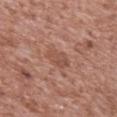Q: Was this lesion biopsied?
A: no biopsy performed (imaged during a skin exam)
Q: What did automated image analysis measure?
A: a mean CIELAB color near L≈51 a*≈23 b*≈28 and a lesion-to-skin contrast of about 5.5 (normalized; higher = more distinct); internal color variation of about 2.5 on a 0–10 scale and peripheral color asymmetry of about 1; a detector confidence of about 100 out of 100 that the crop contains a lesion
Q: What is the imaging modality?
A: total-body-photography crop, ~15 mm field of view
Q: What is the anatomic site?
A: the mid back
Q: Patient demographics?
A: male, aged 43–47
Q: Lesion size?
A: ~3 mm (longest diameter)
Q: Illumination type?
A: white-light illumination